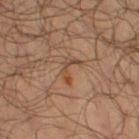workup: total-body-photography surveillance lesion; no biopsy
acquisition: 15 mm crop, total-body photography
tile lighting: cross-polarized
automated metrics: a footprint of about 3 mm²; a mean CIELAB color near L≈43 a*≈19 b*≈29 and a normalized lesion–skin contrast near 6.5; a border-irregularity index near 8.5/10, internal color variation of about 0 on a 0–10 scale, and peripheral color asymmetry of about 0
subject: male, aged around 65
site: the right thigh
lesion diameter: ≈3 mm